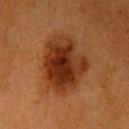{
  "biopsy_status": "not biopsied; imaged during a skin examination",
  "patient": {
    "sex": "female",
    "age_approx": 55
  },
  "lesion_size": {
    "long_diameter_mm_approx": 6.5
  },
  "image": {
    "source": "total-body photography crop",
    "field_of_view_mm": 15
  },
  "site": "left upper arm",
  "lighting": "cross-polarized"
}